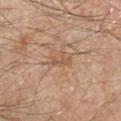The lesion was tiled from a total-body skin photograph and was not biopsied. A close-up tile cropped from a whole-body skin photograph, about 15 mm across. The lesion is on the arm. The patient is a male aged around 45. The lesion-visualizer software estimated a nevus-likeness score of about 0/100 and lesion-presence confidence of about 85/100.